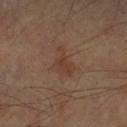Assessment:
Captured during whole-body skin photography for melanoma surveillance; the lesion was not biopsied.
Clinical summary:
A close-up tile cropped from a whole-body skin photograph, about 15 mm across. From the right forearm. A male subject aged approximately 70.